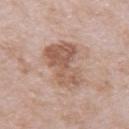The lesion was photographed on a routine skin check and not biopsied; there is no pathology result. A lesion tile, about 15 mm wide, cut from a 3D total-body photograph. Imaged with white-light lighting. A male subject, aged approximately 75. The recorded lesion diameter is about 6 mm. On the left upper arm.